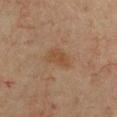follow-up = catalogued during a skin exam; not biopsied
body site = the chest
lesion diameter = ≈3 mm
tile lighting = cross-polarized
subject = female, roughly 40 years of age
automated metrics = a nevus-likeness score of about 0/100 and a lesion-detection confidence of about 100/100
acquisition = ~15 mm tile from a whole-body skin photo